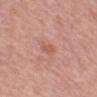Clinical impression:
Recorded during total-body skin imaging; not selected for excision or biopsy.